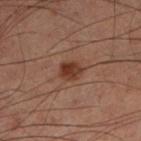workup = imaged on a skin check; not biopsied | patient = male, about 60 years old | lesion size = about 2.5 mm | tile lighting = cross-polarized illumination | image = ~15 mm tile from a whole-body skin photo | body site = the left lower leg.